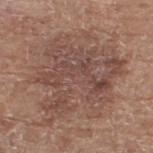Assessment: Part of a total-body skin-imaging series; this lesion was reviewed on a skin check and was not flagged for biopsy. Context: The patient is a female aged approximately 75. Imaged with white-light lighting. From the right thigh. The lesion-visualizer software estimated a lesion color around L≈48 a*≈18 b*≈24 in CIELAB, a lesion–skin lightness drop of about 8, and a normalized lesion–skin contrast near 6. The software also gave a border-irregularity rating of about 7.5/10, a within-lesion color-variation index near 5.5/10, and a peripheral color-asymmetry measure near 2. It also reported an automated nevus-likeness rating near 5 out of 100 and a detector confidence of about 100 out of 100 that the crop contains a lesion. A lesion tile, about 15 mm wide, cut from a 3D total-body photograph.